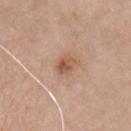Captured during whole-body skin photography for melanoma surveillance; the lesion was not biopsied. The total-body-photography lesion software estimated a footprint of about 4 mm² and an eccentricity of roughly 0.7. The software also gave a lesion color around L≈55 a*≈21 b*≈31 in CIELAB, roughly 10 lightness units darker than nearby skin, and a normalized lesion–skin contrast near 7.5. The analysis additionally found a nevus-likeness score of about 65/100 and a detector confidence of about 100 out of 100 that the crop contains a lesion. A lesion tile, about 15 mm wide, cut from a 3D total-body photograph. From the chest. The tile uses white-light illumination. A male subject aged 73 to 77.